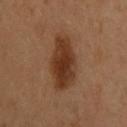– acquisition — ~15 mm tile from a whole-body skin photo
– patient — male, aged around 45
– automated lesion analysis — a footprint of about 17 mm² and an outline eccentricity of about 0.85 (0 = round, 1 = elongated)
– illumination — cross-polarized illumination
– lesion size — about 6.5 mm
– location — the back